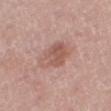– notes: total-body-photography surveillance lesion; no biopsy
– anatomic site: the right thigh
– lighting: white-light
– image: total-body-photography crop, ~15 mm field of view
– TBP lesion metrics: an eccentricity of roughly 0.75 and a symmetry-axis asymmetry near 0.25; a mean CIELAB color near L≈56 a*≈21 b*≈25 and a normalized border contrast of about 6.5; a nevus-likeness score of about 25/100
– patient: male, in their mid- to late 70s